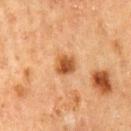This lesion was catalogued during total-body skin photography and was not selected for biopsy. This is a cross-polarized tile. A 15 mm close-up tile from a total-body photography series done for melanoma screening. The lesion is located on the mid back. The total-body-photography lesion software estimated an area of roughly 6 mm², an outline eccentricity of about 0.3 (0 = round, 1 = elongated), and a shape-asymmetry score of about 0.3 (0 = symmetric). The software also gave a border-irregularity index near 2.5/10, internal color variation of about 5 on a 0–10 scale, and a peripheral color-asymmetry measure near 1.5. Measured at roughly 2.5 mm in maximum diameter. A male subject, about 65 years old.